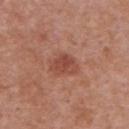Part of a total-body skin-imaging series; this lesion was reviewed on a skin check and was not flagged for biopsy.
A close-up tile cropped from a whole-body skin photograph, about 15 mm across.
Longest diameter approximately 3.5 mm.
The patient is a female roughly 40 years of age.
On the chest.